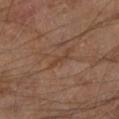{
  "biopsy_status": "not biopsied; imaged during a skin examination",
  "patient": {
    "sex": "male",
    "age_approx": 70
  },
  "image": {
    "source": "total-body photography crop",
    "field_of_view_mm": 15
  },
  "automated_metrics": {
    "eccentricity": 0.95,
    "shape_asymmetry": 0.55,
    "cielab_L": 40,
    "cielab_a": 19,
    "cielab_b": 28,
    "vs_skin_darker_L": 6.0,
    "vs_skin_contrast_norm": 5.5
  },
  "site": "left lower leg",
  "lesion_size": {
    "long_diameter_mm_approx": 3.5
  },
  "lighting": "cross-polarized"
}